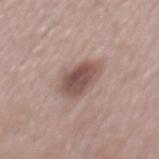biopsy_status: not biopsied; imaged during a skin examination
image:
  source: total-body photography crop
  field_of_view_mm: 15
automated_metrics:
  eccentricity: 0.8
  shape_asymmetry: 0.25
  cielab_L: 50
  cielab_a: 18
  cielab_b: 22
  color_variation_0_10: 4.0
  nevus_likeness_0_100: 65
  lesion_detection_confidence_0_100: 100
patient:
  sex: male
  age_approx: 55
lighting: white-light